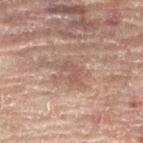{"lesion_size": {"long_diameter_mm_approx": 2.5}, "image": {"source": "total-body photography crop", "field_of_view_mm": 15}, "patient": {"sex": "female", "age_approx": 80}, "site": "right leg"}